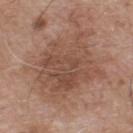Notes:
– biopsy status: imaged on a skin check; not biopsied
– size: ≈7 mm
– patient: male, about 75 years old
– image source: ~15 mm crop, total-body skin-cancer survey
– anatomic site: the chest